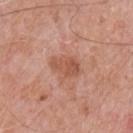The lesion was photographed on a routine skin check and not biopsied; there is no pathology result. A male subject aged approximately 60. Located on the chest. Captured under white-light illumination. Cropped from a whole-body photographic skin survey; the tile spans about 15 mm.